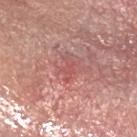biopsy status: total-body-photography surveillance lesion; no biopsy | size: about 3 mm | lighting: white-light | image source: ~15 mm crop, total-body skin-cancer survey | location: the head or neck | patient: male, aged 58 to 62.